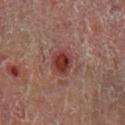follow-up: no biopsy performed (imaged during a skin exam); lesion diameter: about 3 mm; lighting: cross-polarized; subject: male, approximately 55 years of age; location: the left lower leg; imaging modality: ~15 mm tile from a whole-body skin photo.